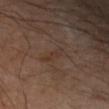Recorded during total-body skin imaging; not selected for excision or biopsy. Captured under cross-polarized illumination. On the right forearm. About 3 mm across. A region of skin cropped from a whole-body photographic capture, roughly 15 mm wide.